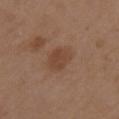Q: What is the anatomic site?
A: the left upper arm
Q: What kind of image is this?
A: ~15 mm crop, total-body skin-cancer survey
Q: What are the patient's age and sex?
A: female, approximately 40 years of age
Q: How large is the lesion?
A: about 2.5 mm
Q: Illumination type?
A: white-light